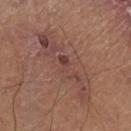Q: Is there a histopathology result?
A: total-body-photography surveillance lesion; no biopsy
Q: How was this image acquired?
A: ~15 mm tile from a whole-body skin photo
Q: What is the anatomic site?
A: the right lower leg
Q: Automated lesion metrics?
A: a border-irregularity index near 7.5/10, a within-lesion color-variation index near 6.5/10, and a peripheral color-asymmetry measure near 2.5; a classifier nevus-likeness of about 0/100 and lesion-presence confidence of about 80/100
Q: Who is the patient?
A: male, approximately 65 years of age